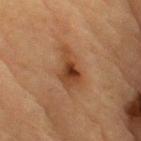The lesion is located on the arm.
A male patient aged 83 or over.
A 15 mm close-up extracted from a 3D total-body photography capture.